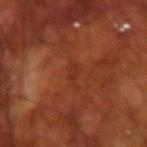biopsy status: no biopsy performed (imaged during a skin exam); anatomic site: the right forearm; image source: total-body-photography crop, ~15 mm field of view; size: ~3 mm (longest diameter); illumination: cross-polarized; patient: male, about 70 years old.